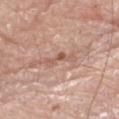On the right thigh. The subject is a male aged approximately 80. A region of skin cropped from a whole-body photographic capture, roughly 15 mm wide.